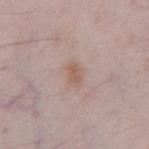workup: catalogued during a skin exam; not biopsied